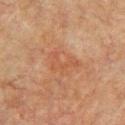Case summary:
* follow-up · imaged on a skin check; not biopsied
* image · ~15 mm crop, total-body skin-cancer survey
* TBP lesion metrics · a mean CIELAB color near L≈44 a*≈21 b*≈30, a lesion–skin lightness drop of about 5, and a lesion-to-skin contrast of about 4.5 (normalized; higher = more distinct)
* subject · male, approximately 75 years of age
* illumination · cross-polarized illumination
* body site · the left upper arm